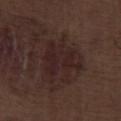follow-up = total-body-photography surveillance lesion; no biopsy
lighting = white-light
subject = male, in their 70s
lesion diameter = ≈5.5 mm
location = the right lower leg
image = 15 mm crop, total-body photography